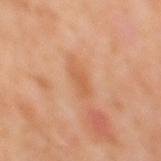Clinical impression:
Captured during whole-body skin photography for melanoma surveillance; the lesion was not biopsied.
Clinical summary:
Cropped from a whole-body photographic skin survey; the tile spans about 15 mm. From the mid back. The lesion's longest dimension is about 4 mm. Imaged with cross-polarized lighting. The patient is a male about 60 years old.